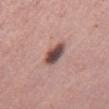Captured during whole-body skin photography for melanoma surveillance; the lesion was not biopsied. A female patient aged 38–42. The lesion is on the right thigh. A 15 mm close-up tile from a total-body photography series done for melanoma screening.